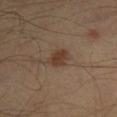Assessment: This lesion was catalogued during total-body skin photography and was not selected for biopsy. Image and clinical context: A lesion tile, about 15 mm wide, cut from a 3D total-body photograph. The tile uses cross-polarized illumination. Longest diameter approximately 3 mm. The patient is a male in their 40s. Automated image analysis of the tile measured a lesion color around L≈38 a*≈16 b*≈27 in CIELAB, roughly 8 lightness units darker than nearby skin, and a normalized border contrast of about 7.5. The lesion is on the left lower leg.